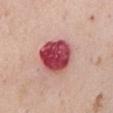Recorded during total-body skin imaging; not selected for excision or biopsy. The total-body-photography lesion software estimated a footprint of about 17 mm², a shape eccentricity near 0.5, and a shape-asymmetry score of about 0.15 (0 = symmetric). A female patient, roughly 65 years of age. About 5 mm across. A roughly 15 mm field-of-view crop from a total-body skin photograph. Located on the chest.